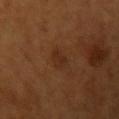Case summary:
- biopsy status: catalogued during a skin exam; not biopsied
- tile lighting: cross-polarized
- image source: total-body-photography crop, ~15 mm field of view
- site: the left upper arm
- lesion diameter: ~2.5 mm (longest diameter)
- patient: female, about 55 years old